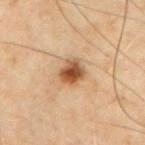The lesion-visualizer software estimated an area of roughly 6.5 mm², an eccentricity of roughly 0.45, and a shape-asymmetry score of about 0.2 (0 = symmetric). The software also gave a lesion–skin lightness drop of about 13. And it measured a border-irregularity index near 2/10 and a peripheral color-asymmetry measure near 1.5. And it measured an automated nevus-likeness rating near 95 out of 100 and a lesion-detection confidence of about 100/100. The subject is a male aged 53 to 57. On the chest. A 15 mm crop from a total-body photograph taken for skin-cancer surveillance. About 3 mm across.